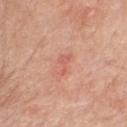Notes:
* biopsy status: total-body-photography surveillance lesion; no biopsy
* size: ~2.5 mm (longest diameter)
* patient: female, approximately 70 years of age
* body site: the chest
* image: ~15 mm tile from a whole-body skin photo
* automated metrics: an area of roughly 2.5 mm² and a symmetry-axis asymmetry near 0.55; a border-irregularity rating of about 6/10 and a color-variation rating of about 0/10; a nevus-likeness score of about 0/100 and a detector confidence of about 100 out of 100 that the crop contains a lesion
* illumination: white-light